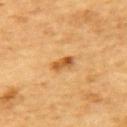notes = catalogued during a skin exam; not biopsied
lesion diameter = ~2.5 mm (longest diameter)
subject = male, roughly 85 years of age or older
body site = the upper back
illumination = cross-polarized illumination
image = ~15 mm crop, total-body skin-cancer survey
image-analysis metrics = a shape eccentricity near 0.85 and a symmetry-axis asymmetry near 0.2; roughly 11 lightness units darker than nearby skin and a normalized lesion–skin contrast near 8.5; a border-irregularity index near 2.5/10 and radial color variation of about 1.5